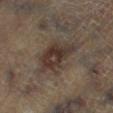Impression: Imaged during a routine full-body skin examination; the lesion was not biopsied and no histopathology is available. Background: A close-up tile cropped from a whole-body skin photograph, about 15 mm across. The lesion is located on the right lower leg. Imaged with cross-polarized lighting. The subject is a male in their mid-60s.